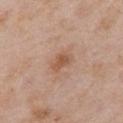This lesion was catalogued during total-body skin photography and was not selected for biopsy. A female subject, aged approximately 70. Cropped from a total-body skin-imaging series; the visible field is about 15 mm. This is a white-light tile. Longest diameter approximately 3 mm. On the right upper arm. Automated tile analysis of the lesion measured an average lesion color of about L≈54 a*≈20 b*≈31 (CIELAB), roughly 9 lightness units darker than nearby skin, and a normalized lesion–skin contrast near 6.5. And it measured a border-irregularity rating of about 3/10, a color-variation rating of about 2.5/10, and a peripheral color-asymmetry measure near 1.